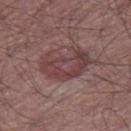{
  "site": "leg",
  "lighting": "white-light",
  "lesion_size": {
    "long_diameter_mm_approx": 6.0
  },
  "automated_metrics": {
    "border_irregularity_0_10": 4.5,
    "color_variation_0_10": 4.0,
    "peripheral_color_asymmetry": 1.0,
    "nevus_likeness_0_100": 35,
    "lesion_detection_confidence_0_100": 95
  },
  "image": {
    "source": "total-body photography crop",
    "field_of_view_mm": 15
  },
  "patient": {
    "sex": "male",
    "age_approx": 65
  }
}